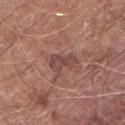biopsy status=imaged on a skin check; not biopsied
image=~15 mm crop, total-body skin-cancer survey
location=the left lower leg
patient=male, in their 80s
lesion diameter=≈3.5 mm
lighting=white-light